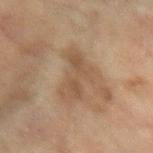The lesion was tiled from a total-body skin photograph and was not biopsied. About 6 mm across. A female patient, roughly 80 years of age. Automated tile analysis of the lesion measured a lesion area of about 12 mm², an outline eccentricity of about 0.9 (0 = round, 1 = elongated), and two-axis asymmetry of about 0.5. It also reported peripheral color asymmetry of about 1. The analysis additionally found a detector confidence of about 95 out of 100 that the crop contains a lesion. Imaged with cross-polarized lighting. The lesion is located on the arm. A 15 mm close-up tile from a total-body photography series done for melanoma screening.